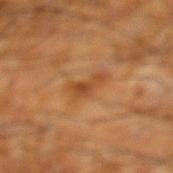Part of a total-body skin-imaging series; this lesion was reviewed on a skin check and was not flagged for biopsy. Automated image analysis of the tile measured a footprint of about 5 mm² and a shape-asymmetry score of about 0.55 (0 = symmetric). The software also gave a border-irregularity index near 5.5/10. The lesion is on the left lower leg. The tile uses cross-polarized illumination. A 15 mm close-up tile from a total-body photography series done for melanoma screening. About 4 mm across. A male subject roughly 60 years of age.